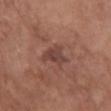Findings:
• patient: female, roughly 75 years of age
• lesion size: ≈3.5 mm
• site: the back
• imaging modality: ~15 mm tile from a whole-body skin photo
• tile lighting: white-light illumination